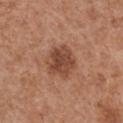workup: catalogued during a skin exam; not biopsied | subject: female, aged around 40 | site: the chest | tile lighting: white-light illumination | imaging modality: ~15 mm tile from a whole-body skin photo.